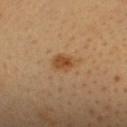biopsy_status: not biopsied; imaged during a skin examination
automated_metrics:
  area_mm2_approx: 5.0
  eccentricity: 0.65
  shape_asymmetry: 0.25
  cielab_L: 50
  cielab_a: 21
  cielab_b: 39
  vs_skin_darker_L: 10.0
  vs_skin_contrast_norm: 8.0
image:
  source: total-body photography crop
  field_of_view_mm: 15
lesion_size:
  long_diameter_mm_approx: 3.0
patient:
  sex: female
  age_approx: 30
site: head or neck